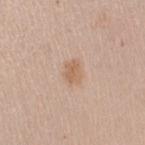Q: Was a biopsy performed?
A: imaged on a skin check; not biopsied
Q: How was the tile lit?
A: white-light illumination
Q: What is the anatomic site?
A: the right upper arm
Q: How was this image acquired?
A: total-body-photography crop, ~15 mm field of view
Q: What are the patient's age and sex?
A: female, in their 40s